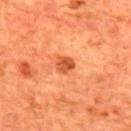* biopsy status · catalogued during a skin exam; not biopsied
* acquisition · ~15 mm crop, total-body skin-cancer survey
* lesion diameter · about 2.5 mm
* patient · male, in their mid-60s
* body site · the mid back
* tile lighting · cross-polarized